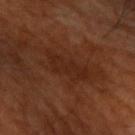No biopsy was performed on this lesion — it was imaged during a full skin examination and was not determined to be concerning. Captured under cross-polarized illumination. The patient is a male aged 58 to 62. About 5 mm across. A 15 mm close-up extracted from a 3D total-body photography capture. On the left upper arm. Automated tile analysis of the lesion measured an eccentricity of roughly 0.85 and a symmetry-axis asymmetry near 0.35. It also reported a border-irregularity index near 4.5/10 and a color-variation rating of about 1.5/10. It also reported an automated nevus-likeness rating near 0 out of 100 and a detector confidence of about 100 out of 100 that the crop contains a lesion.